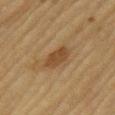{
  "biopsy_status": "not biopsied; imaged during a skin examination",
  "lighting": "cross-polarized",
  "lesion_size": {
    "long_diameter_mm_approx": 3.0
  },
  "image": {
    "source": "total-body photography crop",
    "field_of_view_mm": 15
  },
  "automated_metrics": {
    "area_mm2_approx": 6.0,
    "eccentricity": 0.65
  },
  "patient": {
    "sex": "male",
    "age_approx": "85+"
  },
  "site": "left upper arm"
}